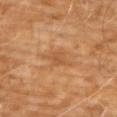Assessment:
The lesion was tiled from a total-body skin photograph and was not biopsied.
Clinical summary:
The lesion-visualizer software estimated an area of roughly 4.5 mm², an eccentricity of roughly 0.85, and two-axis asymmetry of about 0.25. The software also gave peripheral color asymmetry of about 0.5. Imaged with cross-polarized lighting. Approximately 3.5 mm at its widest. On the chest. A male subject, about 60 years old. A region of skin cropped from a whole-body photographic capture, roughly 15 mm wide.